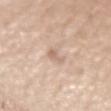Part of a total-body skin-imaging series; this lesion was reviewed on a skin check and was not flagged for biopsy.
Approximately 2.5 mm at its widest.
A 15 mm crop from a total-body photograph taken for skin-cancer surveillance.
On the left upper arm.
This is a white-light tile.
A male subject, about 75 years old.